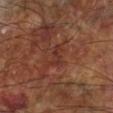Recorded during total-body skin imaging; not selected for excision or biopsy.
From the left lower leg.
A male subject, in their 60s.
A lesion tile, about 15 mm wide, cut from a 3D total-body photograph.
Measured at roughly 3 mm in maximum diameter.